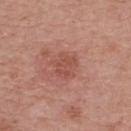Q: Is there a histopathology result?
A: total-body-photography surveillance lesion; no biopsy